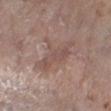Q: Was this lesion biopsied?
A: imaged on a skin check; not biopsied
Q: How was this image acquired?
A: 15 mm crop, total-body photography
Q: Lesion size?
A: ≈6 mm
Q: How was the tile lit?
A: white-light
Q: Where on the body is the lesion?
A: the right lower leg
Q: What are the patient's age and sex?
A: female, in their mid-80s or older